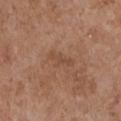{
  "biopsy_status": "not biopsied; imaged during a skin examination",
  "patient": {
    "sex": "female",
    "age_approx": 75
  },
  "site": "chest",
  "image": {
    "source": "total-body photography crop",
    "field_of_view_mm": 15
  },
  "lighting": "white-light",
  "lesion_size": {
    "long_diameter_mm_approx": 3.5
  }
}